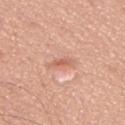workup: imaged on a skin check; not biopsied
lighting: white-light illumination
anatomic site: the upper back
acquisition: total-body-photography crop, ~15 mm field of view
subject: male, in their mid- to late 70s